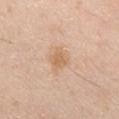Captured during whole-body skin photography for melanoma surveillance; the lesion was not biopsied. The lesion is on the chest. A male subject aged 28–32. Captured under white-light illumination. The total-body-photography lesion software estimated an area of roughly 5 mm², a shape eccentricity near 0.45, and a shape-asymmetry score of about 0.3 (0 = symmetric). The analysis additionally found a mean CIELAB color near L≈65 a*≈18 b*≈35, a lesion–skin lightness drop of about 8, and a normalized lesion–skin contrast near 6. The software also gave an automated nevus-likeness rating near 5 out of 100. This image is a 15 mm lesion crop taken from a total-body photograph.